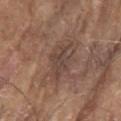follow-up: imaged on a skin check; not biopsied
location: the arm
size: ≈4.5 mm
patient: male, aged 78–82
image: total-body-photography crop, ~15 mm field of view
image-analysis metrics: an area of roughly 9 mm² and a shape eccentricity near 0.8; border irregularity of about 6 on a 0–10 scale, internal color variation of about 3 on a 0–10 scale, and a peripheral color-asymmetry measure near 1; an automated nevus-likeness rating near 0 out of 100 and a lesion-detection confidence of about 100/100
illumination: white-light